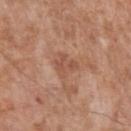Impression:
The lesion was tiled from a total-body skin photograph and was not biopsied.
Acquisition and patient details:
On the left upper arm. Approximately 3 mm at its widest. Automated image analysis of the tile measured a border-irregularity index near 4.5/10 and internal color variation of about 3 on a 0–10 scale. The analysis additionally found an automated nevus-likeness rating near 0 out of 100 and a detector confidence of about 100 out of 100 that the crop contains a lesion. A male subject aged approximately 55. This is a white-light tile. A 15 mm crop from a total-body photograph taken for skin-cancer surveillance.